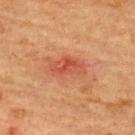Q: How was this image acquired?
A: ~15 mm crop, total-body skin-cancer survey
Q: How large is the lesion?
A: about 4 mm
Q: Illumination type?
A: cross-polarized illumination
Q: Patient demographics?
A: female, in their 70s
Q: What is the anatomic site?
A: the upper back